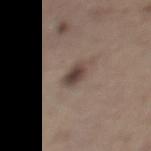Recorded during total-body skin imaging; not selected for excision or biopsy. Located on the left lower leg. Imaged with white-light lighting. A 15 mm close-up tile from a total-body photography series done for melanoma screening. Automated image analysis of the tile measured a lesion color around L≈43 a*≈13 b*≈21 in CIELAB, a lesion–skin lightness drop of about 12, and a normalized border contrast of about 9.5. And it measured a color-variation rating of about 3/10 and radial color variation of about 1. The analysis additionally found an automated nevus-likeness rating near 90 out of 100 and a lesion-detection confidence of about 100/100. The patient is a male aged around 70. Measured at roughly 2.5 mm in maximum diameter.